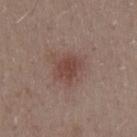patient=male, aged around 30 | body site=the right upper arm | image=total-body-photography crop, ~15 mm field of view | lesion diameter=~3 mm (longest diameter) | tile lighting=white-light illumination.